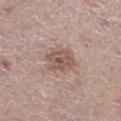This lesion was catalogued during total-body skin photography and was not selected for biopsy.
The recorded lesion diameter is about 4 mm.
A female patient, roughly 50 years of age.
The lesion is located on the left lower leg.
An algorithmic analysis of the crop reported an eccentricity of roughly 0.7 and a symmetry-axis asymmetry near 0.15. The analysis additionally found an average lesion color of about L≈54 a*≈18 b*≈23 (CIELAB), a lesion–skin lightness drop of about 10, and a normalized border contrast of about 7.
The tile uses white-light illumination.
A roughly 15 mm field-of-view crop from a total-body skin photograph.